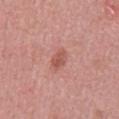This lesion was catalogued during total-body skin photography and was not selected for biopsy. A male subject, aged around 50. Longest diameter approximately 2.5 mm. A roughly 15 mm field-of-view crop from a total-body skin photograph. Located on the abdomen. Captured under white-light illumination. Automated image analysis of the tile measured a mean CIELAB color near L≈54 a*≈27 b*≈27, a lesion–skin lightness drop of about 9, and a normalized border contrast of about 6.5. It also reported border irregularity of about 2 on a 0–10 scale.